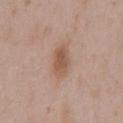site = the chest
image-analysis metrics = a lesion area of about 7 mm² and two-axis asymmetry of about 0.2; an average lesion color of about L≈54 a*≈19 b*≈28 (CIELAB), a lesion–skin lightness drop of about 10, and a normalized lesion–skin contrast near 7
tile lighting = white-light
diameter = ~4 mm (longest diameter)
acquisition = 15 mm crop, total-body photography
patient = male, aged around 50